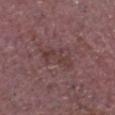biopsy status: imaged on a skin check; not biopsied | site: the head or neck | size: ≈4 mm | patient: male, in their mid-60s | automated lesion analysis: an area of roughly 5 mm², an eccentricity of roughly 0.9, and a symmetry-axis asymmetry near 0.5; a classifier nevus-likeness of about 0/100 | acquisition: total-body-photography crop, ~15 mm field of view.